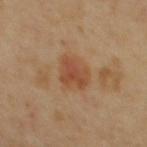Recorded during total-body skin imaging; not selected for excision or biopsy. Captured under cross-polarized illumination. A lesion tile, about 15 mm wide, cut from a 3D total-body photograph. From the upper back. A female patient about 35 years old.